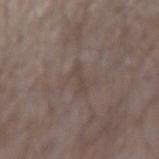automated lesion analysis=a mean CIELAB color near L≈43 a*≈12 b*≈19, about 5 CIELAB-L* units darker than the surrounding skin, and a normalized lesion–skin contrast near 4.5; border irregularity of about 6 on a 0–10 scale, internal color variation of about 0 on a 0–10 scale, and a peripheral color-asymmetry measure near 0
illumination=white-light
patient=male, in their mid- to late 50s
image=15 mm crop, total-body photography
location=the right forearm